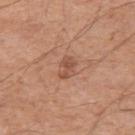Assessment:
Imaged during a routine full-body skin examination; the lesion was not biopsied and no histopathology is available.
Clinical summary:
A male patient in their mid- to late 60s. On the left upper arm. A 15 mm crop from a total-body photograph taken for skin-cancer surveillance.